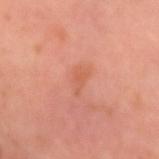The lesion is located on the left forearm.
The subject is a female aged approximately 40.
Longest diameter approximately 3 mm.
A region of skin cropped from a whole-body photographic capture, roughly 15 mm wide.
Imaged with cross-polarized lighting.
An algorithmic analysis of the crop reported a lesion color around L≈59 a*≈28 b*≈34 in CIELAB and a normalized lesion–skin contrast near 4.5. It also reported a border-irregularity index near 4/10 and radial color variation of about 0.5. The analysis additionally found a nevus-likeness score of about 0/100 and a detector confidence of about 100 out of 100 that the crop contains a lesion.